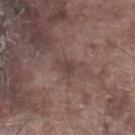This lesion was catalogued during total-body skin photography and was not selected for biopsy.
The lesion-visualizer software estimated internal color variation of about 1 on a 0–10 scale and a peripheral color-asymmetry measure near 0.
A region of skin cropped from a whole-body photographic capture, roughly 15 mm wide.
The lesion is on the leg.
This is a white-light tile.
The recorded lesion diameter is about 2.5 mm.
A male subject, aged 73 to 77.